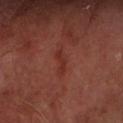workup: imaged on a skin check; not biopsied
lesion size: ≈3 mm
image-analysis metrics: an average lesion color of about L≈29 a*≈27 b*≈26 (CIELAB), about 6 CIELAB-L* units darker than the surrounding skin, and a lesion-to-skin contrast of about 6 (normalized; higher = more distinct); a classifier nevus-likeness of about 5/100 and lesion-presence confidence of about 95/100
patient: male, aged 63–67
site: the left forearm
acquisition: 15 mm crop, total-body photography
lighting: cross-polarized illumination